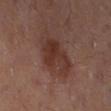| key | value |
|---|---|
| biopsy status | imaged on a skin check; not biopsied |
| TBP lesion metrics | a lesion–skin lightness drop of about 8 and a normalized lesion–skin contrast near 7; a border-irregularity rating of about 3.5/10, a color-variation rating of about 4.5/10, and a peripheral color-asymmetry measure near 1.5 |
| size | about 6 mm |
| anatomic site | the right thigh |
| tile lighting | cross-polarized |
| acquisition | ~15 mm crop, total-body skin-cancer survey |